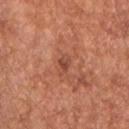lighting = white-light; image = 15 mm crop, total-body photography; patient = male, about 65 years old; location = the chest.